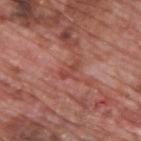Impression: Captured during whole-body skin photography for melanoma surveillance; the lesion was not biopsied. Clinical summary: The patient is a male about 70 years old. An algorithmic analysis of the crop reported a lesion color around L≈46 a*≈27 b*≈28 in CIELAB, roughly 7 lightness units darker than nearby skin, and a normalized lesion–skin contrast near 5.5. It also reported a border-irregularity rating of about 6/10, a color-variation rating of about 0/10, and a peripheral color-asymmetry measure near 0. The analysis additionally found a classifier nevus-likeness of about 0/100. A roughly 15 mm field-of-view crop from a total-body skin photograph. The recorded lesion diameter is about 2.5 mm. From the upper back. Captured under white-light illumination.